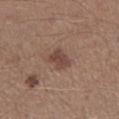biopsy_status: not biopsied; imaged during a skin examination
lighting: white-light
image:
  source: total-body photography crop
  field_of_view_mm: 15
automated_metrics:
  border_irregularity_0_10: 3.5
patient:
  sex: male
  age_approx: 60
site: right lower leg
lesion_size:
  long_diameter_mm_approx: 3.0